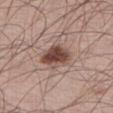A roughly 15 mm field-of-view crop from a total-body skin photograph. The lesion is located on the right thigh. The subject is a male aged 58 to 62.